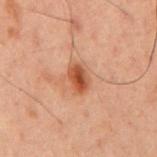{
  "biopsy_status": "not biopsied; imaged during a skin examination",
  "site": "back",
  "automated_metrics": {
    "vs_skin_darker_L": 11.0,
    "vs_skin_contrast_norm": 10.0,
    "border_irregularity_0_10": 2.0,
    "color_variation_0_10": 3.5,
    "peripheral_color_asymmetry": 1.0
  },
  "patient": {
    "sex": "male",
    "age_approx": 60
  },
  "image": {
    "source": "total-body photography crop",
    "field_of_view_mm": 15
  },
  "lesion_size": {
    "long_diameter_mm_approx": 3.0
  }
}